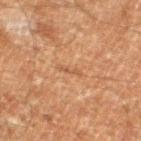Imaged during a routine full-body skin examination; the lesion was not biopsied and no histopathology is available.
From the left lower leg.
This is a cross-polarized tile.
A male subject, in their 60s.
The recorded lesion diameter is about 3 mm.
A region of skin cropped from a whole-body photographic capture, roughly 15 mm wide.